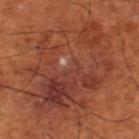Clinical impression: No biopsy was performed on this lesion — it was imaged during a full skin examination and was not determined to be concerning. Image and clinical context: Imaged with cross-polarized lighting. A 15 mm close-up tile from a total-body photography series done for melanoma screening. The lesion's longest dimension is about 12.5 mm. Located on the right lower leg. The lesion-visualizer software estimated a lesion area of about 70 mm². And it measured a mean CIELAB color near L≈41 a*≈27 b*≈30, about 7 CIELAB-L* units darker than the surrounding skin, and a normalized border contrast of about 6.5. The software also gave border irregularity of about 6 on a 0–10 scale, a within-lesion color-variation index near 9/10, and radial color variation of about 3. It also reported a classifier nevus-likeness of about 5/100. A male subject in their mid- to late 60s.